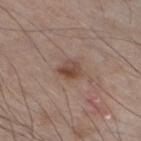  biopsy_status: not biopsied; imaged during a skin examination
  site: left lower leg
  image:
    source: total-body photography crop
    field_of_view_mm: 15
  lighting: white-light
  patient:
    sex: male
    age_approx: 60